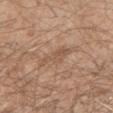Clinical impression: No biopsy was performed on this lesion — it was imaged during a full skin examination and was not determined to be concerning. Background: Located on the right forearm. A male patient, aged approximately 35. Cropped from a whole-body photographic skin survey; the tile spans about 15 mm.